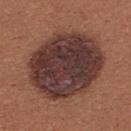No biopsy was performed on this lesion — it was imaged during a full skin examination and was not determined to be concerning. From the upper back. Automated image analysis of the tile measured a lesion area of about 55 mm² and two-axis asymmetry of about 0.1. The analysis additionally found a nevus-likeness score of about 0/100 and a lesion-detection confidence of about 100/100. The recorded lesion diameter is about 9 mm. Cropped from a whole-body photographic skin survey; the tile spans about 15 mm. A female patient, in their mid-20s. Imaged with white-light lighting.